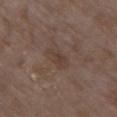Part of a total-body skin-imaging series; this lesion was reviewed on a skin check and was not flagged for biopsy. The lesion is on the chest. Cropped from a whole-body photographic skin survey; the tile spans about 15 mm. The lesion-visualizer software estimated a lesion color around L≈39 a*≈15 b*≈23 in CIELAB, about 6 CIELAB-L* units darker than the surrounding skin, and a normalized border contrast of about 5.5. The software also gave a classifier nevus-likeness of about 0/100 and lesion-presence confidence of about 100/100. About 3.5 mm across. Imaged with white-light lighting. A female subject aged 83 or over.